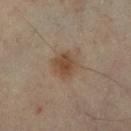workup: no biopsy performed (imaged during a skin exam); lesion diameter: ≈3 mm; illumination: cross-polarized; subject: male, approximately 55 years of age; imaging modality: 15 mm crop, total-body photography; body site: the right lower leg.